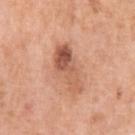Recorded during total-body skin imaging; not selected for excision or biopsy. The patient is a female aged 68–72. Approximately 6 mm at its widest. A lesion tile, about 15 mm wide, cut from a 3D total-body photograph. Captured under white-light illumination. From the left upper arm.